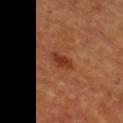biopsy status — total-body-photography surveillance lesion; no biopsy
patient — male, aged approximately 50
illumination — cross-polarized
image-analysis metrics — a footprint of about 4.5 mm² and an eccentricity of roughly 0.85; about 9 CIELAB-L* units darker than the surrounding skin and a normalized lesion–skin contrast near 7.5; a border-irregularity index near 2.5/10, a within-lesion color-variation index near 2.5/10, and radial color variation of about 0.5; a classifier nevus-likeness of about 85/100
acquisition — 15 mm crop, total-body photography
site — the front of the torso